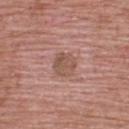| field | value |
|---|---|
| workup | imaged on a skin check; not biopsied |
| patient | male, aged 48–52 |
| acquisition | ~15 mm tile from a whole-body skin photo |
| tile lighting | white-light |
| location | the back |